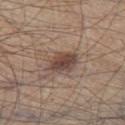Recorded during total-body skin imaging; not selected for excision or biopsy. A lesion tile, about 15 mm wide, cut from a 3D total-body photograph. On the leg. Automated image analysis of the tile measured a shape eccentricity near 0.7. It also reported border irregularity of about 2 on a 0–10 scale and internal color variation of about 4 on a 0–10 scale. And it measured an automated nevus-likeness rating near 90 out of 100 and a lesion-detection confidence of about 100/100. A male subject, roughly 45 years of age. Imaged with white-light lighting.